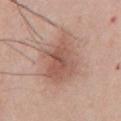Case summary:
* biopsy status: catalogued during a skin exam; not biopsied
* anatomic site: the chest
* tile lighting: white-light illumination
* subject: male, roughly 55 years of age
* image source: ~15 mm tile from a whole-body skin photo